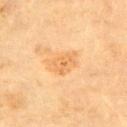- biopsy status: total-body-photography surveillance lesion; no biopsy
- illumination: cross-polarized illumination
- diameter: about 4 mm
- subject: male, approximately 85 years of age or older
- acquisition: ~15 mm tile from a whole-body skin photo
- automated lesion analysis: a lesion area of about 7.5 mm², an eccentricity of roughly 0.75, and a symmetry-axis asymmetry near 0.3
- anatomic site: the chest